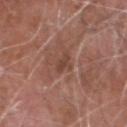Imaged during a routine full-body skin examination; the lesion was not biopsied and no histopathology is available.
A roughly 15 mm field-of-view crop from a total-body skin photograph.
Longest diameter approximately 2.5 mm.
A male subject aged approximately 75.
From the head or neck.
This is a white-light tile.